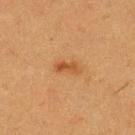notes = total-body-photography surveillance lesion; no biopsy
acquisition = 15 mm crop, total-body photography
patient = female, approximately 40 years of age
site = the left lower leg